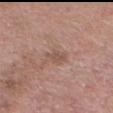Background:
The lesion is on the head or neck. A male patient, aged 63–67. Approximately 2.5 mm at its widest. This is a white-light tile. A 15 mm close-up extracted from a 3D total-body photography capture. The total-body-photography lesion software estimated a mean CIELAB color near L≈52 a*≈19 b*≈24, a lesion–skin lightness drop of about 7, and a normalized lesion–skin contrast near 5. And it measured internal color variation of about 1.5 on a 0–10 scale and peripheral color asymmetry of about 1.